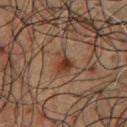Clinical impression: The lesion was photographed on a routine skin check and not biopsied; there is no pathology result. Clinical summary: The total-body-photography lesion software estimated an average lesion color of about L≈28 a*≈16 b*≈24 (CIELAB), a lesion–skin lightness drop of about 7, and a normalized lesion–skin contrast near 8. The analysis additionally found an automated nevus-likeness rating near 75 out of 100 and lesion-presence confidence of about 100/100. A male subject aged approximately 60. The lesion is on the chest. A 15 mm crop from a total-body photograph taken for skin-cancer surveillance.